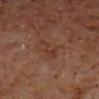notes: total-body-photography surveillance lesion; no biopsy | location: the right lower leg | subject: male, about 60 years old | lighting: cross-polarized illumination | image source: ~15 mm crop, total-body skin-cancer survey.